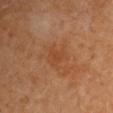Assessment:
Captured during whole-body skin photography for melanoma surveillance; the lesion was not biopsied.
Background:
From the left upper arm. Automated tile analysis of the lesion measured a lesion area of about 1.5 mm², an outline eccentricity of about 0.7 (0 = round, 1 = elongated), and two-axis asymmetry of about 0.2. It also reported a mean CIELAB color near L≈43 a*≈25 b*≈36, a lesion–skin lightness drop of about 4, and a normalized border contrast of about 4. The analysis additionally found a border-irregularity rating of about 1.5/10, a color-variation rating of about 0/10, and peripheral color asymmetry of about 0. Captured under cross-polarized illumination. A 15 mm close-up tile from a total-body photography series done for melanoma screening. A female patient, about 50 years old. The lesion's longest dimension is about 1.5 mm.